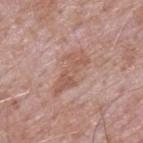workup: catalogued during a skin exam; not biopsied
tile lighting: white-light illumination
automated lesion analysis: lesion-presence confidence of about 100/100
location: the upper back
patient: male, roughly 65 years of age
acquisition: ~15 mm crop, total-body skin-cancer survey
size: about 6 mm